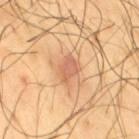Imaged during a routine full-body skin examination; the lesion was not biopsied and no histopathology is available. The lesion is located on the right thigh. A 15 mm close-up tile from a total-body photography series done for melanoma screening. The subject is a male in their mid-60s. An algorithmic analysis of the crop reported a lesion area of about 5 mm² and an outline eccentricity of about 0.75 (0 = round, 1 = elongated). It also reported a mean CIELAB color near L≈64 a*≈25 b*≈36, a lesion–skin lightness drop of about 10, and a lesion-to-skin contrast of about 6.5 (normalized; higher = more distinct). The analysis additionally found a nevus-likeness score of about 15/100 and a detector confidence of about 100 out of 100 that the crop contains a lesion. The tile uses cross-polarized illumination.